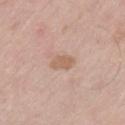Case summary:
– notes · no biopsy performed (imaged during a skin exam)
– location · the left thigh
– subject · male, in their 60s
– tile lighting · white-light illumination
– acquisition · 15 mm crop, total-body photography
– lesion size · ≈3 mm
– automated lesion analysis · border irregularity of about 2 on a 0–10 scale, a within-lesion color-variation index near 2/10, and peripheral color asymmetry of about 1